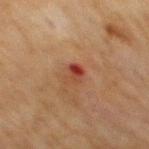Clinical impression:
Captured during whole-body skin photography for melanoma surveillance; the lesion was not biopsied.
Acquisition and patient details:
The lesion-visualizer software estimated a classifier nevus-likeness of about 0/100. On the mid back. A region of skin cropped from a whole-body photographic capture, roughly 15 mm wide. Imaged with cross-polarized lighting. A male subject approximately 70 years of age.